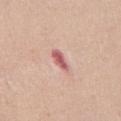follow-up = no biopsy performed (imaged during a skin exam) | subject = female, in their mid-40s | location = the abdomen | lesion size = about 3 mm | TBP lesion metrics = a border-irregularity rating of about 3/10, a within-lesion color-variation index near 2/10, and peripheral color asymmetry of about 0.5; a nevus-likeness score of about 0/100 and a lesion-detection confidence of about 100/100 | imaging modality = 15 mm crop, total-body photography.